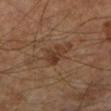biopsy status: total-body-photography surveillance lesion; no biopsy
patient: male, aged around 65
imaging modality: ~15 mm tile from a whole-body skin photo
tile lighting: cross-polarized illumination
lesion size: ~3 mm (longest diameter)